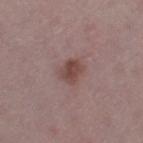The lesion was photographed on a routine skin check and not biopsied; there is no pathology result. The lesion is on the left thigh. The patient is a female approximately 45 years of age. A 15 mm crop from a total-body photograph taken for skin-cancer surveillance.